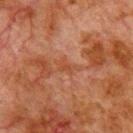| key | value |
|---|---|
| biopsy status | no biopsy performed (imaged during a skin exam) |
| body site | the chest |
| tile lighting | cross-polarized illumination |
| image-analysis metrics | a lesion area of about 3.5 mm², an eccentricity of roughly 0.8, and a shape-asymmetry score of about 0.55 (0 = symmetric); roughly 5 lightness units darker than nearby skin; an automated nevus-likeness rating near 0 out of 100 and a lesion-detection confidence of about 80/100 |
| subject | male, roughly 80 years of age |
| image source | total-body-photography crop, ~15 mm field of view |